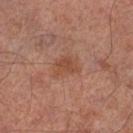subject = male, aged around 65; location = the left thigh; lesion diameter = ≈3.5 mm; image source = ~15 mm tile from a whole-body skin photo.